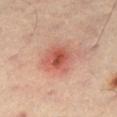follow-up: catalogued during a skin exam; not biopsied | subject: male, aged 53–57 | automated metrics: an area of roughly 11 mm², an outline eccentricity of about 0.35 (0 = round, 1 = elongated), and two-axis asymmetry of about 0.15; a lesion color around L≈54 a*≈28 b*≈29 in CIELAB and a lesion–skin lightness drop of about 11; a border-irregularity rating of about 1.5/10, internal color variation of about 5.5 on a 0–10 scale, and a peripheral color-asymmetry measure near 1.5 | image source: ~15 mm tile from a whole-body skin photo | site: the right thigh | illumination: cross-polarized.